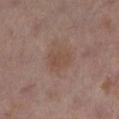The lesion was photographed on a routine skin check and not biopsied; there is no pathology result.
Captured under cross-polarized illumination.
The lesion-visualizer software estimated an average lesion color of about L≈46 a*≈18 b*≈25 (CIELAB), a lesion–skin lightness drop of about 6, and a lesion-to-skin contrast of about 5.5 (normalized; higher = more distinct).
From the left lower leg.
A female subject in their 40s.
A close-up tile cropped from a whole-body skin photograph, about 15 mm across.